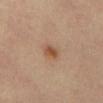Impression:
The lesion was photographed on a routine skin check and not biopsied; there is no pathology result.
Background:
A male patient, aged 63 to 67. Automated image analysis of the tile measured a lesion area of about 3.5 mm² and a shape-asymmetry score of about 0.2 (0 = symmetric). And it measured border irregularity of about 2 on a 0–10 scale and internal color variation of about 2.5 on a 0–10 scale. It also reported a nevus-likeness score of about 95/100 and a lesion-detection confidence of about 100/100. A 15 mm close-up tile from a total-body photography series done for melanoma screening. Approximately 2 mm at its widest. Located on the abdomen.